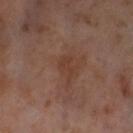Assessment:
The lesion was tiled from a total-body skin photograph and was not biopsied.
Clinical summary:
A female subject, aged 53–57. A 15 mm close-up extracted from a 3D total-body photography capture. Longest diameter approximately 2.5 mm. Imaged with cross-polarized lighting. Located on the left thigh.